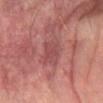Notes:
– biopsy status: imaged on a skin check; not biopsied
– lighting: cross-polarized illumination
– subject: male, aged around 75
– image source: 15 mm crop, total-body photography
– diameter: ≈3.5 mm
– body site: the right forearm
– image-analysis metrics: an area of roughly 8.5 mm² and two-axis asymmetry of about 0.2; about 6 CIELAB-L* units darker than the surrounding skin and a normalized lesion–skin contrast near 5; internal color variation of about 2.5 on a 0–10 scale and a peripheral color-asymmetry measure near 1